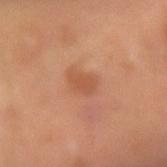| feature | finding |
|---|---|
| notes | total-body-photography surveillance lesion; no biopsy |
| subject | male, approximately 65 years of age |
| diameter | about 2.5 mm |
| imaging modality | ~15 mm crop, total-body skin-cancer survey |
| image-analysis metrics | an outline eccentricity of about 0.75 (0 = round, 1 = elongated); a mean CIELAB color near L≈51 a*≈25 b*≈35, about 7 CIELAB-L* units darker than the surrounding skin, and a lesion-to-skin contrast of about 5.5 (normalized; higher = more distinct); border irregularity of about 2 on a 0–10 scale and a within-lesion color-variation index near 1/10; a nevus-likeness score of about 20/100 |
| lighting | cross-polarized illumination |